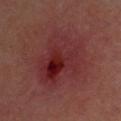notes — no biopsy performed (imaged during a skin exam)
patient — male, in their mid-60s
body site — the head or neck
size — about 7.5 mm
acquisition — 15 mm crop, total-body photography